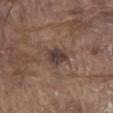Notes:
• biopsy status: imaged on a skin check; not biopsied
• size: ≈2.5 mm
• automated metrics: a footprint of about 5.5 mm² and two-axis asymmetry of about 0.25; a lesion–skin lightness drop of about 10 and a lesion-to-skin contrast of about 9.5 (normalized; higher = more distinct); border irregularity of about 2.5 on a 0–10 scale, a within-lesion color-variation index near 3/10, and a peripheral color-asymmetry measure near 1; a detector confidence of about 100 out of 100 that the crop contains a lesion
• patient: male, aged approximately 80
• anatomic site: the abdomen
• image: ~15 mm crop, total-body skin-cancer survey